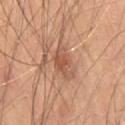Clinical impression: Part of a total-body skin-imaging series; this lesion was reviewed on a skin check and was not flagged for biopsy. Context: Longest diameter approximately 4 mm. Cropped from a whole-body photographic skin survey; the tile spans about 15 mm. The lesion is on the right thigh. The patient is a male aged around 70. Captured under cross-polarized illumination.